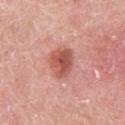The lesion was tiled from a total-body skin photograph and was not biopsied. A male subject aged 78–82. A roughly 15 mm field-of-view crop from a total-body skin photograph. The tile uses white-light illumination. Automated tile analysis of the lesion measured an area of roughly 9 mm², a shape eccentricity near 0.65, and a symmetry-axis asymmetry near 0.2. The software also gave an average lesion color of about L≈55 a*≈29 b*≈28 (CIELAB) and a normalized border contrast of about 8.5. It also reported a border-irregularity rating of about 2.5/10 and a within-lesion color-variation index near 4/10. And it measured a nevus-likeness score of about 95/100 and lesion-presence confidence of about 100/100. Located on the left upper arm.